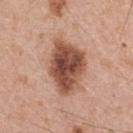No biopsy was performed on this lesion — it was imaged during a full skin examination and was not determined to be concerning. Located on the chest. Cropped from a whole-body photographic skin survey; the tile spans about 15 mm. A male subject, aged approximately 55.